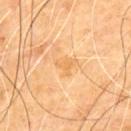<lesion>
<biopsy_status>not biopsied; imaged during a skin examination</biopsy_status>
<site>upper back</site>
<image>
  <source>total-body photography crop</source>
  <field_of_view_mm>15</field_of_view_mm>
</image>
<lighting>cross-polarized</lighting>
<automated_metrics>
  <area_mm2_approx>4.0</area_mm2_approx>
  <shape_asymmetry>0.5</shape_asymmetry>
  <cielab_L>70</cielab_L>
  <cielab_a>21</cielab_a>
  <cielab_b>47</cielab_b>
  <lesion_detection_confidence_0_100>100</lesion_detection_confidence_0_100>
</automated_metrics>
<patient>
  <sex>male</sex>
  <age_approx>70</age_approx>
</patient>
</lesion>